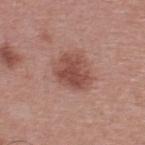Clinical summary:
The tile uses white-light illumination. The lesion is on the upper back. A male patient about 40 years old. This image is a 15 mm lesion crop taken from a total-body photograph.